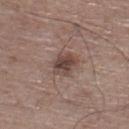<case>
<biopsy_status>not biopsied; imaged during a skin examination</biopsy_status>
<site>right thigh</site>
<lesion_size>
  <long_diameter_mm_approx>3.5</long_diameter_mm_approx>
</lesion_size>
<image>
  <source>total-body photography crop</source>
  <field_of_view_mm>15</field_of_view_mm>
</image>
<lighting>white-light</lighting>
<patient>
  <sex>male</sex>
  <age_approx>65</age_approx>
</patient>
</case>